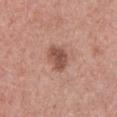biopsy status = no biopsy performed (imaged during a skin exam) | imaging modality = ~15 mm tile from a whole-body skin photo | location = the chest | subject = male, aged approximately 60 | tile lighting = white-light illumination | lesion diameter = about 4 mm.